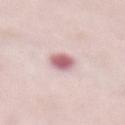Impression: Imaged during a routine full-body skin examination; the lesion was not biopsied and no histopathology is available. Context: The lesion is on the abdomen. Captured under white-light illumination. The lesion's longest dimension is about 3 mm. A lesion tile, about 15 mm wide, cut from a 3D total-body photograph. A female subject roughly 65 years of age.